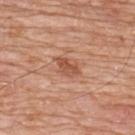tile lighting=white-light | TBP lesion metrics=a mean CIELAB color near L≈53 a*≈25 b*≈32, roughly 10 lightness units darker than nearby skin, and a normalized lesion–skin contrast near 7; a classifier nevus-likeness of about 20/100 | image source=15 mm crop, total-body photography | body site=the upper back | lesion size=≈3.5 mm | patient=male, aged 78–82.